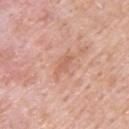tile lighting=white-light; size=about 3 mm; site=the upper back; subject=male, roughly 55 years of age; acquisition=total-body-photography crop, ~15 mm field of view.